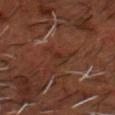<record>
  <biopsy_status>not biopsied; imaged during a skin examination</biopsy_status>
</record>